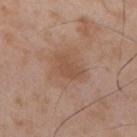Clinical impression:
Captured during whole-body skin photography for melanoma surveillance; the lesion was not biopsied.
Background:
A male patient aged 48 to 52. On the back. A close-up tile cropped from a whole-body skin photograph, about 15 mm across.